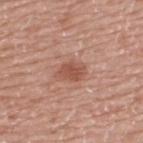Recorded during total-body skin imaging; not selected for excision or biopsy.
A region of skin cropped from a whole-body photographic capture, roughly 15 mm wide.
Measured at roughly 3.5 mm in maximum diameter.
The lesion is located on the back.
A male subject in their mid-70s.